Captured under white-light illumination. A male patient, roughly 50 years of age. A 15 mm close-up extracted from a 3D total-body photography capture. Located on the right thigh. Approximately 5 mm at its widest — 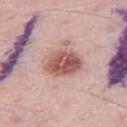– histopathologic diagnosis · a dysplastic (Clark) nevus